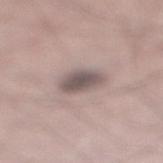The lesion was tiled from a total-body skin photograph and was not biopsied. This is a white-light tile. Measured at roughly 3.5 mm in maximum diameter. A male patient in their mid- to late 30s. The total-body-photography lesion software estimated an area of roughly 8 mm², a shape eccentricity near 0.7, and a symmetry-axis asymmetry near 0.15. It also reported roughly 13 lightness units darker than nearby skin and a lesion-to-skin contrast of about 9 (normalized; higher = more distinct). And it measured border irregularity of about 1.5 on a 0–10 scale, a within-lesion color-variation index near 3/10, and a peripheral color-asymmetry measure near 1. The analysis additionally found a classifier nevus-likeness of about 5/100 and a lesion-detection confidence of about 95/100. From the right lower leg. A region of skin cropped from a whole-body photographic capture, roughly 15 mm wide.